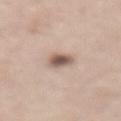biopsy_status: not biopsied; imaged during a skin examination
site: abdomen
lesion_size:
  long_diameter_mm_approx: 2.5
automated_metrics:
  area_mm2_approx: 5.0
  eccentricity: 0.55
  shape_asymmetry: 0.25
patient:
  sex: male
  age_approx: 60
image:
  source: total-body photography crop
  field_of_view_mm: 15